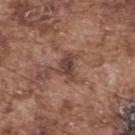The lesion was photographed on a routine skin check and not biopsied; there is no pathology result. Automated image analysis of the tile measured an area of roughly 5 mm². The software also gave a lesion color around L≈41 a*≈20 b*≈23 in CIELAB, roughly 10 lightness units darker than nearby skin, and a lesion-to-skin contrast of about 8.5 (normalized; higher = more distinct). It also reported an automated nevus-likeness rating near 5 out of 100. A male patient aged 73–77. The tile uses white-light illumination. Approximately 3.5 mm at its widest. A 15 mm crop from a total-body photograph taken for skin-cancer surveillance. The lesion is located on the upper back.